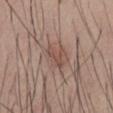Part of a total-body skin-imaging series; this lesion was reviewed on a skin check and was not flagged for biopsy. The subject is a male roughly 50 years of age. From the abdomen. Cropped from a total-body skin-imaging series; the visible field is about 15 mm.